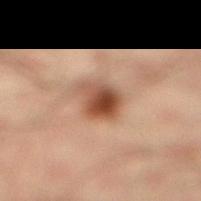biopsy status — catalogued during a skin exam; not biopsied
subject — male, approximately 35 years of age
location — the right lower leg
lesion diameter — about 4 mm
automated lesion analysis — an area of roughly 8.5 mm², an outline eccentricity of about 0.65 (0 = round, 1 = elongated), and a symmetry-axis asymmetry near 0.25; a border-irregularity rating of about 2.5/10 and a color-variation rating of about 9/10; lesion-presence confidence of about 100/100
acquisition — 15 mm crop, total-body photography